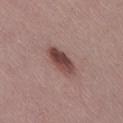Impression: The lesion was photographed on a routine skin check and not biopsied; there is no pathology result. Image and clinical context: Imaged with white-light lighting. The lesion is on the right thigh. The subject is a female aged approximately 45. A roughly 15 mm field-of-view crop from a total-body skin photograph. The total-body-photography lesion software estimated a footprint of about 8 mm² and two-axis asymmetry of about 0.2. The analysis additionally found a color-variation rating of about 6/10 and peripheral color asymmetry of about 2.5. The recorded lesion diameter is about 4.5 mm.